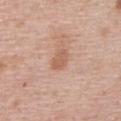follow-up: total-body-photography surveillance lesion; no biopsy
image source: total-body-photography crop, ~15 mm field of view
subject: female, aged 48 to 52
tile lighting: white-light illumination
size: ~2.5 mm (longest diameter)
body site: the upper back
automated lesion analysis: an area of roughly 4 mm² and an outline eccentricity of about 0.7 (0 = round, 1 = elongated); a lesion color around L≈59 a*≈21 b*≈31 in CIELAB, about 8 CIELAB-L* units darker than the surrounding skin, and a normalized lesion–skin contrast near 6; an automated nevus-likeness rating near 20 out of 100 and a lesion-detection confidence of about 100/100